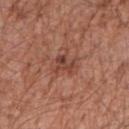Findings:
* biopsy status · imaged on a skin check; not biopsied
* illumination · white-light
* patient · male, in their mid-50s
* size · ≈2.5 mm
* automated metrics · a lesion color around L≈43 a*≈24 b*≈27 in CIELAB, about 9 CIELAB-L* units darker than the surrounding skin, and a normalized border contrast of about 7; an automated nevus-likeness rating near 5 out of 100 and a detector confidence of about 100 out of 100 that the crop contains a lesion
* image · ~15 mm crop, total-body skin-cancer survey
* location · the right forearm